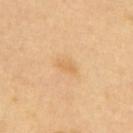biopsy status = total-body-photography surveillance lesion; no biopsy
body site = the back
subject = female, about 60 years old
lighting = cross-polarized
image source = ~15 mm crop, total-body skin-cancer survey